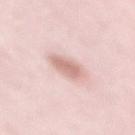Background:
A 15 mm crop from a total-body photograph taken for skin-cancer surveillance. A female patient, aged around 40. The lesion is located on the back.